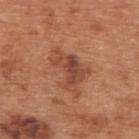Imaged during a routine full-body skin examination; the lesion was not biopsied and no histopathology is available.
The tile uses white-light illumination.
The patient is a male about 65 years old.
An algorithmic analysis of the crop reported a lesion–skin lightness drop of about 10 and a lesion-to-skin contrast of about 7 (normalized; higher = more distinct). The analysis additionally found a border-irregularity rating of about 7/10 and a peripheral color-asymmetry measure near 1.
A roughly 15 mm field-of-view crop from a total-body skin photograph.
On the back.